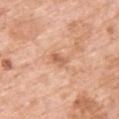biopsy status: total-body-photography surveillance lesion; no biopsy